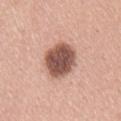Assessment:
Part of a total-body skin-imaging series; this lesion was reviewed on a skin check and was not flagged for biopsy.
Context:
Approximately 5 mm at its widest. A region of skin cropped from a whole-body photographic capture, roughly 15 mm wide. This is a white-light tile. The lesion is on the lower back. Automated image analysis of the tile measured a lesion area of about 15 mm² and a shape-asymmetry score of about 0.1 (0 = symmetric). The software also gave a lesion–skin lightness drop of about 19. The subject is a female aged 28–32.